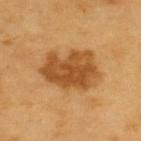Notes:
- size — ~6.5 mm (longest diameter)
- TBP lesion metrics — an eccentricity of roughly 0.75 and a symmetry-axis asymmetry near 0.2; a mean CIELAB color near L≈50 a*≈23 b*≈43 and a lesion-to-skin contrast of about 9 (normalized; higher = more distinct); a border-irregularity index near 3/10 and radial color variation of about 1.5
- location — the upper back
- patient — male, aged around 60
- imaging modality — total-body-photography crop, ~15 mm field of view